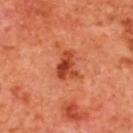<record>
  <biopsy_status>not biopsied; imaged during a skin examination</biopsy_status>
  <patient>
    <sex>female</sex>
    <age_approx>40</age_approx>
  </patient>
  <site>upper back</site>
  <lesion_size>
    <long_diameter_mm_approx>3.0</long_diameter_mm_approx>
  </lesion_size>
  <lighting>cross-polarized</lighting>
  <automated_metrics>
    <nevus_likeness_0_100>75</nevus_likeness_0_100>
    <lesion_detection_confidence_0_100>100</lesion_detection_confidence_0_100>
  </automated_metrics>
  <image>
    <source>total-body photography crop</source>
    <field_of_view_mm>15</field_of_view_mm>
  </image>
</record>